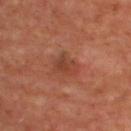No biopsy was performed on this lesion — it was imaged during a full skin examination and was not determined to be concerning. The lesion is on the upper back. The subject is a female about 45 years old. A region of skin cropped from a whole-body photographic capture, roughly 15 mm wide. Imaged with cross-polarized lighting.